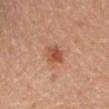{"biopsy_status": "not biopsied; imaged during a skin examination", "image": {"source": "total-body photography crop", "field_of_view_mm": 15}, "automated_metrics": {"area_mm2_approx": 5.0, "eccentricity": 0.75, "shape_asymmetry": 0.2, "color_variation_0_10": 4.5, "peripheral_color_asymmetry": 1.5, "lesion_detection_confidence_0_100": 100}, "lighting": "cross-polarized", "site": "arm", "lesion_size": {"long_diameter_mm_approx": 2.5}, "patient": {"sex": "female", "age_approx": 45}}